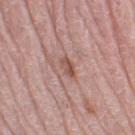Impression: Captured during whole-body skin photography for melanoma surveillance; the lesion was not biopsied. Background: A lesion tile, about 15 mm wide, cut from a 3D total-body photograph. The lesion is located on the left leg. The subject is a female aged approximately 65.